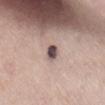<record>
  <biopsy_status>not biopsied; imaged during a skin examination</biopsy_status>
</record>